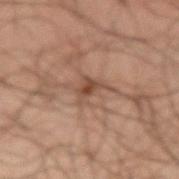Clinical impression: This lesion was catalogued during total-body skin photography and was not selected for biopsy. Acquisition and patient details: The lesion-visualizer software estimated a footprint of about 5.5 mm², an outline eccentricity of about 0.6 (0 = round, 1 = elongated), and a symmetry-axis asymmetry near 0.55. It also reported an average lesion color of about L≈35 a*≈14 b*≈22 (CIELAB) and a lesion–skin lightness drop of about 7. The analysis additionally found a classifier nevus-likeness of about 10/100 and a detector confidence of about 85 out of 100 that the crop contains a lesion. Captured under cross-polarized illumination. Located on the left forearm. A region of skin cropped from a whole-body photographic capture, roughly 15 mm wide. The patient is a male aged around 50.